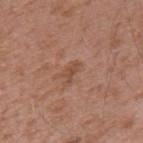Q: Was this lesion biopsied?
A: catalogued during a skin exam; not biopsied
Q: How was the tile lit?
A: white-light
Q: What kind of image is this?
A: total-body-photography crop, ~15 mm field of view
Q: How large is the lesion?
A: ≈2.5 mm
Q: Lesion location?
A: the upper back
Q: Who is the patient?
A: male, aged approximately 45